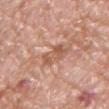{
  "patient": {
    "sex": "male",
    "age_approx": 55
  },
  "image": {
    "source": "total-body photography crop",
    "field_of_view_mm": 15
  },
  "lesion_size": {
    "long_diameter_mm_approx": 3.5
  },
  "lighting": "white-light",
  "site": "chest"
}